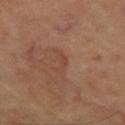* biopsy status — imaged on a skin check; not biopsied
* lesion diameter — ~2.5 mm (longest diameter)
* automated lesion analysis — about 5 CIELAB-L* units darker than the surrounding skin and a normalized lesion–skin contrast near 5; a classifier nevus-likeness of about 5/100 and a lesion-detection confidence of about 90/100
* anatomic site — the right leg
* image source — ~15 mm tile from a whole-body skin photo
* subject — female, about 60 years old